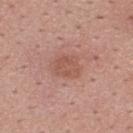The subject is a male aged 23–27. Cropped from a total-body skin-imaging series; the visible field is about 15 mm. From the upper back. The tile uses white-light illumination. Longest diameter approximately 3 mm.